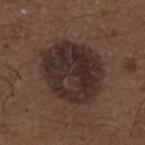Part of a total-body skin-imaging series; this lesion was reviewed on a skin check and was not flagged for biopsy. Measured at roughly 8 mm in maximum diameter. The lesion-visualizer software estimated an area of roughly 36 mm², an eccentricity of roughly 0.65, and a symmetry-axis asymmetry near 0.15. It also reported a mean CIELAB color near L≈28 a*≈15 b*≈19, roughly 10 lightness units darker than nearby skin, and a normalized lesion–skin contrast near 11. It also reported border irregularity of about 1.5 on a 0–10 scale and internal color variation of about 5.5 on a 0–10 scale. The subject is a male aged 48–52. Imaged with white-light lighting. Located on the upper back. A 15 mm close-up extracted from a 3D total-body photography capture.